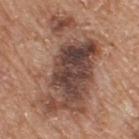image source: ~15 mm crop, total-body skin-cancer survey
diameter: ~12 mm (longest diameter)
location: the upper back
illumination: white-light
subject: male, roughly 65 years of age